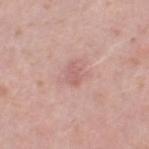{
  "biopsy_status": "not biopsied; imaged during a skin examination",
  "image": {
    "source": "total-body photography crop",
    "field_of_view_mm": 15
  },
  "patient": {
    "sex": "female",
    "age_approx": 40
  },
  "automated_metrics": {
    "area_mm2_approx": 3.0,
    "eccentricity": 0.8
  },
  "lesion_size": {
    "long_diameter_mm_approx": 2.5
  },
  "lighting": "white-light",
  "site": "leg"
}